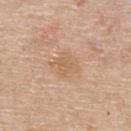| field | value |
|---|---|
| notes | total-body-photography surveillance lesion; no biopsy |
| lesion diameter | ≈3.5 mm |
| imaging modality | ~15 mm tile from a whole-body skin photo |
| site | the upper back |
| patient | male, in their mid-50s |